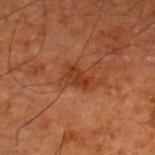Notes:
• workup — imaged on a skin check; not biopsied
• subject — male, aged around 80
• location — the right thigh
• diameter — ~3 mm (longest diameter)
• image-analysis metrics — an area of roughly 4 mm², an eccentricity of roughly 0.8, and two-axis asymmetry of about 0.3; a lesion color around L≈28 a*≈21 b*≈29 in CIELAB, about 7 CIELAB-L* units darker than the surrounding skin, and a normalized lesion–skin contrast near 7.5; border irregularity of about 3 on a 0–10 scale; a classifier nevus-likeness of about 0/100 and lesion-presence confidence of about 100/100
• image — ~15 mm crop, total-body skin-cancer survey